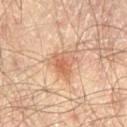| field | value |
|---|---|
| notes | catalogued during a skin exam; not biopsied |
| image source | 15 mm crop, total-body photography |
| TBP lesion metrics | an area of roughly 8 mm², an eccentricity of roughly 0.45, and a symmetry-axis asymmetry near 0.45; an average lesion color of about L≈62 a*≈22 b*≈34 (CIELAB) and roughly 10 lightness units darker than nearby skin; a border-irregularity rating of about 4.5/10, internal color variation of about 5 on a 0–10 scale, and peripheral color asymmetry of about 1.5; a classifier nevus-likeness of about 40/100 and lesion-presence confidence of about 100/100 |
| lesion diameter | about 3.5 mm |
| anatomic site | the left thigh |
| patient | male, roughly 65 years of age |